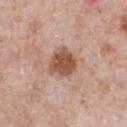biopsy_status: not biopsied; imaged during a skin examination
image:
  source: total-body photography crop
  field_of_view_mm: 15
site: abdomen
patient:
  sex: male
  age_approx: 60
lesion_size:
  long_diameter_mm_approx: 3.5
lighting: white-light